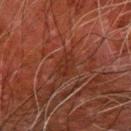<record>
  <biopsy_status>not biopsied; imaged during a skin examination</biopsy_status>
  <image>
    <source>total-body photography crop</source>
    <field_of_view_mm>15</field_of_view_mm>
  </image>
  <lighting>cross-polarized</lighting>
  <site>left forearm</site>
  <patient>
    <sex>male</sex>
    <age_approx>80</age_approx>
  </patient>
  <lesion_size>
    <long_diameter_mm_approx>3.0</long_diameter_mm_approx>
  </lesion_size>
</record>